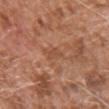Q: What is the anatomic site?
A: the chest
Q: Lesion size?
A: ~2.5 mm (longest diameter)
Q: What lighting was used for the tile?
A: white-light
Q: What is the imaging modality?
A: ~15 mm crop, total-body skin-cancer survey
Q: Patient demographics?
A: male, approximately 75 years of age